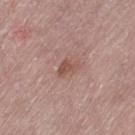biopsy_status: not biopsied; imaged during a skin examination
lighting: white-light
lesion_size:
  long_diameter_mm_approx: 2.5
patient:
  sex: female
  age_approx: 65
image:
  source: total-body photography crop
  field_of_view_mm: 15
site: leg
automated_metrics:
  area_mm2_approx: 4.0
  eccentricity: 0.7
  shape_asymmetry: 0.35
  cielab_L: 52
  cielab_a: 22
  cielab_b: 25
  vs_skin_darker_L: 8.0
  vs_skin_contrast_norm: 6.5
  nevus_likeness_0_100: 30
  lesion_detection_confidence_0_100: 100